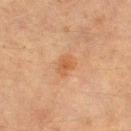Imaged during a routine full-body skin examination; the lesion was not biopsied and no histopathology is available. This is a cross-polarized tile. The subject is a female about 55 years old. From the left thigh. A roughly 15 mm field-of-view crop from a total-body skin photograph.